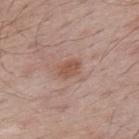biopsy_status: not biopsied; imaged during a skin examination
lesion_size:
  long_diameter_mm_approx: 3.0
image:
  source: total-body photography crop
  field_of_view_mm: 15
automated_metrics:
  border_irregularity_0_10: 1.5
  color_variation_0_10: 1.5
  peripheral_color_asymmetry: 0.5
  nevus_likeness_0_100: 5
  lesion_detection_confidence_0_100: 100
lighting: white-light
site: back
patient:
  sex: male
  age_approx: 65